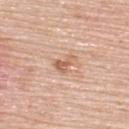{
  "biopsy_status": "not biopsied; imaged during a skin examination",
  "patient": {
    "sex": "female",
    "age_approx": 65
  },
  "lesion_size": {
    "long_diameter_mm_approx": 3.0
  },
  "site": "upper back",
  "image": {
    "source": "total-body photography crop",
    "field_of_view_mm": 15
  },
  "lighting": "white-light"
}